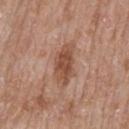Assessment: Captured during whole-body skin photography for melanoma surveillance; the lesion was not biopsied.